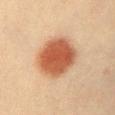| field | value |
|---|---|
| subject | male, in their 40s |
| image source | 15 mm crop, total-body photography |
| location | the arm |
| illumination | cross-polarized illumination |